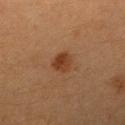Recorded during total-body skin imaging; not selected for excision or biopsy.
Located on the right upper arm.
A 15 mm close-up tile from a total-body photography series done for melanoma screening.
The subject is a female aged 38–42.